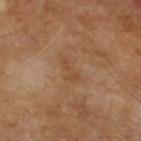Clinical summary:
About 3.5 mm across. The tile uses cross-polarized illumination. A male patient, about 65 years old. Automated tile analysis of the lesion measured an area of roughly 3 mm², an outline eccentricity of about 0.95 (0 = round, 1 = elongated), and a symmetry-axis asymmetry near 0.5. A 15 mm crop from a total-body photograph taken for skin-cancer surveillance.